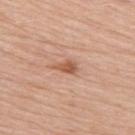<record>
<biopsy_status>not biopsied; imaged during a skin examination</biopsy_status>
<site>right upper arm</site>
<automated_metrics>
  <area_mm2_approx>3.0</area_mm2_approx>
  <eccentricity>0.8</eccentricity>
  <shape_asymmetry>0.3</shape_asymmetry>
  <border_irregularity_0_10>3.0</border_irregularity_0_10>
  <color_variation_0_10>2.5</color_variation_0_10>
  <peripheral_color_asymmetry>1.0</peripheral_color_asymmetry>
</automated_metrics>
<lighting>white-light</lighting>
<lesion_size>
  <long_diameter_mm_approx>2.5</long_diameter_mm_approx>
</lesion_size>
<image>
  <source>total-body photography crop</source>
  <field_of_view_mm>15</field_of_view_mm>
</image>
<patient>
  <sex>female</sex>
  <age_approx>50</age_approx>
</patient>
</record>